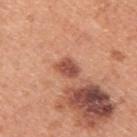Recorded during total-body skin imaging; not selected for excision or biopsy. A region of skin cropped from a whole-body photographic capture, roughly 15 mm wide. Imaged with white-light lighting. The lesion-visualizer software estimated an eccentricity of roughly 0.7 and a shape-asymmetry score of about 0.25 (0 = symmetric). And it measured a lesion-to-skin contrast of about 9 (normalized; higher = more distinct). The analysis additionally found a color-variation rating of about 3/10. The lesion is located on the upper back. Longest diameter approximately 3 mm. A male subject aged 43–47.